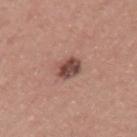Q: Is there a histopathology result?
A: total-body-photography surveillance lesion; no biopsy
Q: What are the patient's age and sex?
A: male, aged around 40
Q: What lighting was used for the tile?
A: white-light illumination
Q: What is the imaging modality?
A: ~15 mm crop, total-body skin-cancer survey
Q: Automated lesion metrics?
A: a lesion area of about 6 mm² and two-axis asymmetry of about 0.15; a mean CIELAB color near L≈47 a*≈21 b*≈23 and roughly 14 lightness units darker than nearby skin; a lesion-detection confidence of about 100/100
Q: What is the lesion's diameter?
A: about 3 mm
Q: Lesion location?
A: the left upper arm